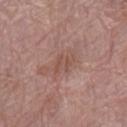{"biopsy_status": "not biopsied; imaged during a skin examination", "patient": {"sex": "female", "age_approx": 70}, "image": {"source": "total-body photography crop", "field_of_view_mm": 15}, "lesion_size": {"long_diameter_mm_approx": 2.5}, "automated_metrics": {"area_mm2_approx": 4.0, "shape_asymmetry": 0.3, "cielab_L": 50, "cielab_a": 20, "cielab_b": 25, "vs_skin_darker_L": 7.0, "border_irregularity_0_10": 4.0, "peripheral_color_asymmetry": 1.0}, "site": "right thigh", "lighting": "white-light"}